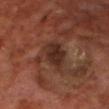notes: total-body-photography surveillance lesion; no biopsy
lighting: cross-polarized
diameter: about 4.5 mm
subject: male, about 70 years old
anatomic site: the chest
imaging modality: ~15 mm crop, total-body skin-cancer survey
automated lesion analysis: a lesion area of about 10 mm², an outline eccentricity of about 0.75 (0 = round, 1 = elongated), and two-axis asymmetry of about 0.25; a border-irregularity index near 3/10, a within-lesion color-variation index near 3.5/10, and a peripheral color-asymmetry measure near 1